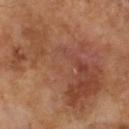notes: catalogued during a skin exam; not biopsied | lighting: cross-polarized illumination | diameter: about 12 mm | image source: ~15 mm crop, total-body skin-cancer survey | image-analysis metrics: internal color variation of about 6.5 on a 0–10 scale | subject: male, approximately 65 years of age.